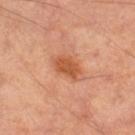anatomic site: the leg
subject: female
acquisition: total-body-photography crop, ~15 mm field of view